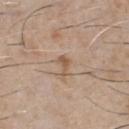The lesion was photographed on a routine skin check and not biopsied; there is no pathology result. The subject is a male roughly 60 years of age. Imaged with white-light lighting. A 15 mm close-up tile from a total-body photography series done for melanoma screening. An algorithmic analysis of the crop reported a lesion color around L≈56 a*≈16 b*≈30 in CIELAB and a normalized lesion–skin contrast near 6.5. The analysis additionally found a border-irregularity index near 4.5/10, internal color variation of about 1 on a 0–10 scale, and radial color variation of about 0.5. The lesion is located on the front of the torso. The lesion's longest dimension is about 2.5 mm.